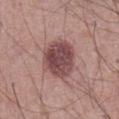The lesion is located on the front of the torso.
A male subject aged approximately 70.
This image is a 15 mm lesion crop taken from a total-body photograph.
Histopathological examination showed a dysplastic (Clark) nevus.